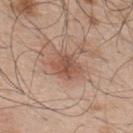Case summary:
* notes · imaged on a skin check; not biopsied
* image-analysis metrics · a mean CIELAB color near L≈52 a*≈21 b*≈29 and about 10 CIELAB-L* units darker than the surrounding skin; border irregularity of about 2.5 on a 0–10 scale, a color-variation rating of about 3.5/10, and peripheral color asymmetry of about 1
* lighting · white-light
* image source · 15 mm crop, total-body photography
* subject · male, roughly 50 years of age
* site · the upper back